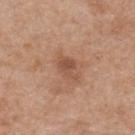- biopsy status · no biopsy performed (imaged during a skin exam)
- image source · 15 mm crop, total-body photography
- illumination · white-light illumination
- anatomic site · the back
- lesion size · ≈3 mm
- subject · female, approximately 75 years of age